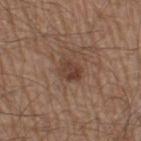Captured during whole-body skin photography for melanoma surveillance; the lesion was not biopsied.
An algorithmic analysis of the crop reported an outline eccentricity of about 0.65 (0 = round, 1 = elongated) and two-axis asymmetry of about 0.2. The software also gave a mean CIELAB color near L≈40 a*≈18 b*≈26, about 8 CIELAB-L* units darker than the surrounding skin, and a lesion-to-skin contrast of about 7 (normalized; higher = more distinct). The software also gave a classifier nevus-likeness of about 20/100 and lesion-presence confidence of about 100/100.
The subject is a male aged around 60.
The lesion is on the left thigh.
Longest diameter approximately 3 mm.
A lesion tile, about 15 mm wide, cut from a 3D total-body photograph.